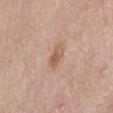Captured during whole-body skin photography for melanoma surveillance; the lesion was not biopsied.
Cropped from a whole-body photographic skin survey; the tile spans about 15 mm.
Captured under white-light illumination.
Measured at roughly 3 mm in maximum diameter.
Automated image analysis of the tile measured a border-irregularity rating of about 2.5/10, internal color variation of about 4 on a 0–10 scale, and radial color variation of about 1.5. And it measured an automated nevus-likeness rating near 5 out of 100 and a lesion-detection confidence of about 100/100.
The patient is a male aged around 80.
Located on the mid back.